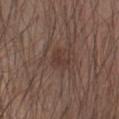Q: Is there a histopathology result?
A: total-body-photography surveillance lesion; no biopsy
Q: What lighting was used for the tile?
A: white-light
Q: What is the anatomic site?
A: the right forearm
Q: What are the patient's age and sex?
A: male, aged approximately 45
Q: What is the lesion's diameter?
A: ≈2.5 mm
Q: Automated lesion metrics?
A: a footprint of about 4 mm², a shape eccentricity near 0.75, and a symmetry-axis asymmetry near 0.45; a mean CIELAB color near L≈36 a*≈17 b*≈22, roughly 5 lightness units darker than nearby skin, and a normalized border contrast of about 5; a border-irregularity rating of about 4.5/10 and radial color variation of about 0.5
Q: What is the imaging modality?
A: total-body-photography crop, ~15 mm field of view